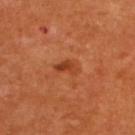follow-up: catalogued during a skin exam; not biopsied
patient: female, approximately 55 years of age
lesion size: about 3 mm
tile lighting: cross-polarized illumination
image: total-body-photography crop, ~15 mm field of view
location: the upper back
automated lesion analysis: a border-irregularity index near 3.5/10, a color-variation rating of about 4.5/10, and peripheral color asymmetry of about 1.5